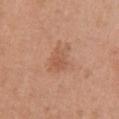follow-up: catalogued during a skin exam; not biopsied
lesion size: ~3 mm (longest diameter)
lighting: white-light illumination
anatomic site: the chest
imaging modality: ~15 mm crop, total-body skin-cancer survey
patient: female, roughly 65 years of age
TBP lesion metrics: a lesion area of about 6.5 mm², an outline eccentricity of about 0.65 (0 = round, 1 = elongated), and a shape-asymmetry score of about 0.35 (0 = symmetric); border irregularity of about 4 on a 0–10 scale, a within-lesion color-variation index near 2.5/10, and peripheral color asymmetry of about 1